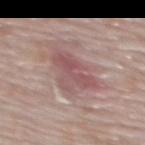Impression:
Imaged during a routine full-body skin examination; the lesion was not biopsied and no histopathology is available.
Background:
The lesion is located on the upper back. The tile uses white-light illumination. The patient is a female aged 63 to 67. The lesion-visualizer software estimated a lesion area of about 13 mm², an eccentricity of roughly 0.65, and two-axis asymmetry of about 0.45. The analysis additionally found roughly 9 lightness units darker than nearby skin and a normalized border contrast of about 6. About 5.5 mm across. A close-up tile cropped from a whole-body skin photograph, about 15 mm across.